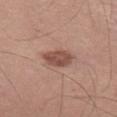Image and clinical context: A male subject aged approximately 55. Imaged with white-light lighting. The lesion is on the left thigh. The lesion's longest dimension is about 4 mm. A lesion tile, about 15 mm wide, cut from a 3D total-body photograph. An algorithmic analysis of the crop reported a footprint of about 7.5 mm². It also reported a lesion–skin lightness drop of about 12 and a normalized border contrast of about 8.5. The analysis additionally found a border-irregularity rating of about 2/10, internal color variation of about 3 on a 0–10 scale, and peripheral color asymmetry of about 1. The software also gave a lesion-detection confidence of about 100/100.